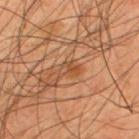The lesion was photographed on a routine skin check and not biopsied; there is no pathology result. Measured at roughly 2.5 mm in maximum diameter. The lesion is on the upper back. A male subject, aged 43–47. A lesion tile, about 15 mm wide, cut from a 3D total-body photograph.